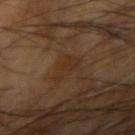notes=total-body-photography surveillance lesion; no biopsy | illumination=cross-polarized | subject=male, roughly 65 years of age | body site=the right upper arm | lesion diameter=about 3.5 mm | image=~15 mm crop, total-body skin-cancer survey | TBP lesion metrics=a border-irregularity index near 6/10 and radial color variation of about 0.5; a lesion-detection confidence of about 100/100.